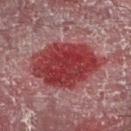notes — total-body-photography surveillance lesion; no biopsy
tile lighting — cross-polarized
acquisition — ~15 mm tile from a whole-body skin photo
subject — male, approximately 55 years of age
size — ≈8.5 mm
site — the right lower leg